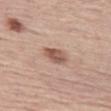Assessment: Recorded during total-body skin imaging; not selected for excision or biopsy. Background: A male patient in their mid- to late 70s. About 3 mm across. Cropped from a whole-body photographic skin survey; the tile spans about 15 mm. From the left thigh. This is a white-light tile.